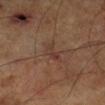{
  "biopsy_status": "not biopsied; imaged during a skin examination",
  "lighting": "cross-polarized",
  "patient": {
    "sex": "male",
    "age_approx": 65
  },
  "site": "left leg",
  "lesion_size": {
    "long_diameter_mm_approx": 3.5
  },
  "image": {
    "source": "total-body photography crop",
    "field_of_view_mm": 15
  }
}